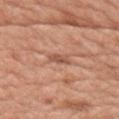The lesion was tiled from a total-body skin photograph and was not biopsied.
The total-body-photography lesion software estimated an area of roughly 3 mm², an outline eccentricity of about 0.85 (0 = round, 1 = elongated), and a symmetry-axis asymmetry near 0.3. The software also gave a border-irregularity rating of about 3/10, a within-lesion color-variation index near 2.5/10, and peripheral color asymmetry of about 1.
This image is a 15 mm lesion crop taken from a total-body photograph.
Measured at roughly 2.5 mm in maximum diameter.
The tile uses white-light illumination.
From the right upper arm.
A male patient aged 58–62.